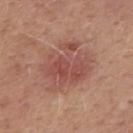Q: Was this lesion biopsied?
A: no biopsy performed (imaged during a skin exam)
Q: Who is the patient?
A: male, roughly 50 years of age
Q: What did automated image analysis measure?
A: a shape eccentricity near 0.6; about 8 CIELAB-L* units darker than the surrounding skin and a normalized lesion–skin contrast near 6.5; a border-irregularity index near 3.5/10 and internal color variation of about 5 on a 0–10 scale; a classifier nevus-likeness of about 60/100 and a detector confidence of about 100 out of 100 that the crop contains a lesion
Q: Lesion location?
A: the mid back
Q: How was this image acquired?
A: ~15 mm crop, total-body skin-cancer survey